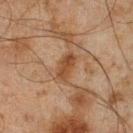imaging modality = 15 mm crop, total-body photography
tile lighting = cross-polarized
site = the leg
subject = male, roughly 45 years of age
lesion diameter = ≈3.5 mm